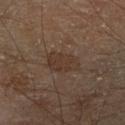  biopsy_status: not biopsied; imaged during a skin examination
  automated_metrics:
    border_irregularity_0_10: 2.5
    color_variation_0_10: 2.5
    nevus_likeness_0_100: 0
    lesion_detection_confidence_0_100: 100
  image:
    source: total-body photography crop
    field_of_view_mm: 15
  patient:
    sex: male
    age_approx: 70
  lesion_size:
    long_diameter_mm_approx: 4.5
  site: right lower leg